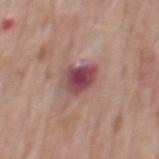biopsy_status: not biopsied; imaged during a skin examination
image:
  source: total-body photography crop
  field_of_view_mm: 15
patient:
  sex: male
  age_approx: 75
automated_metrics:
  area_mm2_approx: 8.5
  eccentricity: 0.5
  shape_asymmetry: 0.25
  border_irregularity_0_10: 2.5
  color_variation_0_10: 5.5
  nevus_likeness_0_100: 0
lesion_size:
  long_diameter_mm_approx: 3.5
site: back